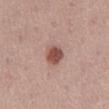Notes:
• biopsy status — total-body-photography surveillance lesion; no biopsy
• body site — the abdomen
• image — total-body-photography crop, ~15 mm field of view
• subject — male, aged 53 to 57
• lighting — white-light
• lesion diameter — ≈3 mm